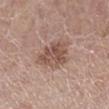The lesion is on the right lower leg. The recorded lesion diameter is about 4 mm. A female subject about 50 years old. A 15 mm crop from a total-body photograph taken for skin-cancer surveillance. Captured under white-light illumination. An algorithmic analysis of the crop reported a lesion area of about 9.5 mm². The software also gave an average lesion color of about L≈51 a*≈19 b*≈25 (CIELAB), roughly 10 lightness units darker than nearby skin, and a normalized lesion–skin contrast near 7.5. And it measured a border-irregularity rating of about 3.5/10, internal color variation of about 4.5 on a 0–10 scale, and a peripheral color-asymmetry measure near 1.5. It also reported a nevus-likeness score of about 10/100.